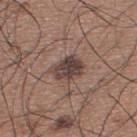follow-up: catalogued during a skin exam; not biopsied
imaging modality: ~15 mm tile from a whole-body skin photo
body site: the upper back
subject: male, in their mid- to late 30s